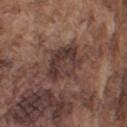<tbp_lesion>
<biopsy_status>not biopsied; imaged during a skin examination</biopsy_status>
<image>
  <source>total-body photography crop</source>
  <field_of_view_mm>15</field_of_view_mm>
</image>
<patient>
  <sex>male</sex>
  <age_approx>75</age_approx>
</patient>
<site>left upper arm</site>
</tbp_lesion>